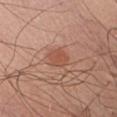| key | value |
|---|---|
| biopsy status | no biopsy performed (imaged during a skin exam) |
| subject | male, aged 38–42 |
| location | the front of the torso |
| size | ~2.5 mm (longest diameter) |
| lighting | white-light illumination |
| image-analysis metrics | a lesion area of about 5 mm², an outline eccentricity of about 0.45 (0 = round, 1 = elongated), and a shape-asymmetry score of about 0.25 (0 = symmetric); a border-irregularity index near 2/10 |
| image source | ~15 mm tile from a whole-body skin photo |